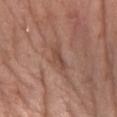Q: Was a biopsy performed?
A: total-body-photography surveillance lesion; no biopsy
Q: What did automated image analysis measure?
A: a shape-asymmetry score of about 0.4 (0 = symmetric); a lesion color around L≈46 a*≈21 b*≈27 in CIELAB, a lesion–skin lightness drop of about 8, and a lesion-to-skin contrast of about 6 (normalized; higher = more distinct)
Q: What is the anatomic site?
A: the arm
Q: What lighting was used for the tile?
A: white-light
Q: Patient demographics?
A: female, about 70 years old
Q: What kind of image is this?
A: ~15 mm tile from a whole-body skin photo